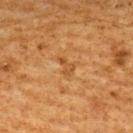Longest diameter approximately 2.5 mm. Automated tile analysis of the lesion measured a lesion color around L≈43 a*≈21 b*≈38 in CIELAB, roughly 6 lightness units darker than nearby skin, and a normalized border contrast of about 5.5. It also reported internal color variation of about 0.5 on a 0–10 scale and radial color variation of about 0. The software also gave lesion-presence confidence of about 100/100. Captured under cross-polarized illumination. The subject is a male aged around 60. On the upper back. Cropped from a total-body skin-imaging series; the visible field is about 15 mm.